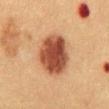Q: Is there a histopathology result?
A: total-body-photography surveillance lesion; no biopsy
Q: What kind of image is this?
A: total-body-photography crop, ~15 mm field of view
Q: Illumination type?
A: cross-polarized illumination
Q: Automated lesion metrics?
A: a lesion area of about 20 mm² and an eccentricity of roughly 0.6; a lesion-to-skin contrast of about 12 (normalized; higher = more distinct); internal color variation of about 5 on a 0–10 scale and radial color variation of about 1.5; a nevus-likeness score of about 100/100 and lesion-presence confidence of about 100/100
Q: Who is the patient?
A: male, in their 50s
Q: Lesion location?
A: the abdomen
Q: How large is the lesion?
A: about 5.5 mm